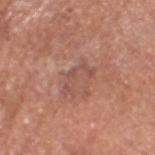The lesion was tiled from a total-body skin photograph and was not biopsied. Approximately 4 mm at its widest. On the head or neck. A male subject in their mid- to late 60s. A 15 mm crop from a total-body photograph taken for skin-cancer surveillance.